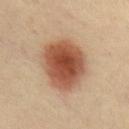Case summary:
- biopsy status — no biopsy performed (imaged during a skin exam)
- image source — ~15 mm crop, total-body skin-cancer survey
- subject — male, aged 38 to 42
- location — the chest